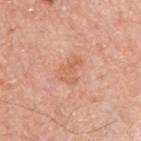Part of a total-body skin-imaging series; this lesion was reviewed on a skin check and was not flagged for biopsy. A male subject, roughly 80 years of age. Approximately 3.5 mm at its widest. A 15 mm crop from a total-body photograph taken for skin-cancer surveillance. This is a white-light tile. The lesion is located on the right upper arm.